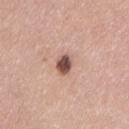biopsy status — total-body-photography surveillance lesion; no biopsy
site — the leg
subject — female, approximately 35 years of age
imaging modality — ~15 mm crop, total-body skin-cancer survey
image-analysis metrics — a lesion area of about 4 mm² and an eccentricity of roughly 0.7; a mean CIELAB color near L≈51 a*≈20 b*≈25, roughly 18 lightness units darker than nearby skin, and a lesion-to-skin contrast of about 12 (normalized; higher = more distinct); a border-irregularity index near 1.5/10 and peripheral color asymmetry of about 1.5; an automated nevus-likeness rating near 90 out of 100
lesion diameter — about 2.5 mm